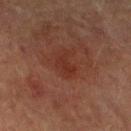biopsy_status: not biopsied; imaged during a skin examination